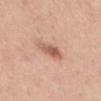Clinical impression: Part of a total-body skin-imaging series; this lesion was reviewed on a skin check and was not flagged for biopsy.